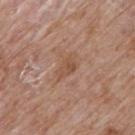Recorded during total-body skin imaging; not selected for excision or biopsy.